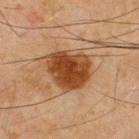Clinical impression:
Imaged during a routine full-body skin examination; the lesion was not biopsied and no histopathology is available.
Context:
A male patient, approximately 45 years of age. The total-body-photography lesion software estimated a border-irregularity index near 2/10, a within-lesion color-variation index near 4.5/10, and peripheral color asymmetry of about 1.5. Longest diameter approximately 5.5 mm. A lesion tile, about 15 mm wide, cut from a 3D total-body photograph. Located on the upper back.